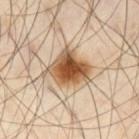- biopsy status — total-body-photography surveillance lesion; no biopsy
- lighting — cross-polarized illumination
- site — the left thigh
- subject — male, in their mid-50s
- imaging modality — 15 mm crop, total-body photography
- lesion size — ~5 mm (longest diameter)